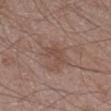Imaged during a routine full-body skin examination; the lesion was not biopsied and no histopathology is available.
Measured at roughly 3 mm in maximum diameter.
Automated image analysis of the tile measured a lesion area of about 4.5 mm² and a shape eccentricity near 0.75. The software also gave internal color variation of about 1 on a 0–10 scale.
A male patient, aged approximately 60.
A 15 mm crop from a total-body photograph taken for skin-cancer surveillance.
On the right lower leg.
The tile uses white-light illumination.